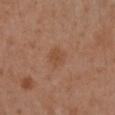Impression: Recorded during total-body skin imaging; not selected for excision or biopsy. Image and clinical context: On the left arm. The subject is a female about 40 years old. A close-up tile cropped from a whole-body skin photograph, about 15 mm across.